An algorithmic analysis of the crop reported internal color variation of about 3 on a 0–10 scale and peripheral color asymmetry of about 1. The analysis additionally found a nevus-likeness score of about 0/100 and a lesion-detection confidence of about 95/100 · longest diameter approximately 4 mm · a male subject about 60 years old · on the head or neck · a close-up tile cropped from a whole-body skin photograph, about 15 mm across:
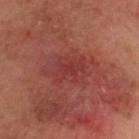Findings:
• diagnosis: a lichen planus-like keratosis